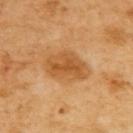{"biopsy_status": "not biopsied; imaged during a skin examination", "site": "upper back", "lesion_size": {"long_diameter_mm_approx": 5.5}, "automated_metrics": {"lesion_detection_confidence_0_100": 100}, "image": {"source": "total-body photography crop", "field_of_view_mm": 15}, "patient": {"sex": "female", "age_approx": 55}, "lighting": "cross-polarized"}